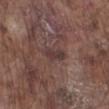Part of a total-body skin-imaging series; this lesion was reviewed on a skin check and was not flagged for biopsy. Located on the right lower leg. A male patient, approximately 75 years of age. A roughly 15 mm field-of-view crop from a total-body skin photograph.